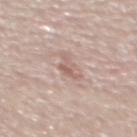Recorded during total-body skin imaging; not selected for excision or biopsy.
On the upper back.
This image is a 15 mm lesion crop taken from a total-body photograph.
A male subject, aged 58–62.